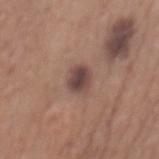follow-up: no biopsy performed (imaged during a skin exam)
lesion size: ~3 mm (longest diameter)
image-analysis metrics: a lesion area of about 6 mm², an outline eccentricity of about 0.7 (0 = round, 1 = elongated), and two-axis asymmetry of about 0.2
acquisition: ~15 mm tile from a whole-body skin photo
subject: male, aged 63 to 67
anatomic site: the mid back
illumination: white-light